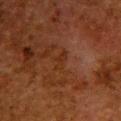Part of a total-body skin-imaging series; this lesion was reviewed on a skin check and was not flagged for biopsy. Approximately 2.5 mm at its widest. Cropped from a whole-body photographic skin survey; the tile spans about 15 mm. Automated image analysis of the tile measured an area of roughly 3 mm². It also reported roughly 4 lightness units darker than nearby skin and a normalized lesion–skin contrast near 5.5. And it measured a detector confidence of about 100 out of 100 that the crop contains a lesion. The patient is a female about 50 years old. The lesion is on the upper back. This is a cross-polarized tile.